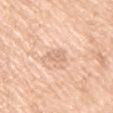No biopsy was performed on this lesion — it was imaged during a full skin examination and was not determined to be concerning. This is a white-light tile. Cropped from a total-body skin-imaging series; the visible field is about 15 mm. The patient is a female aged approximately 55. The lesion is on the chest.